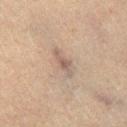No biopsy was performed on this lesion — it was imaged during a full skin examination and was not determined to be concerning. Captured under cross-polarized illumination. A 15 mm close-up tile from a total-body photography series done for melanoma screening. A male subject, aged 63–67. Automated tile analysis of the lesion measured a border-irregularity index near 4/10, a within-lesion color-variation index near 1.5/10, and radial color variation of about 0.5. On the leg. Longest diameter approximately 3.5 mm.